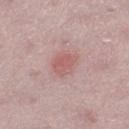Assessment:
Imaged during a routine full-body skin examination; the lesion was not biopsied and no histopathology is available.
Background:
The total-body-photography lesion software estimated a footprint of about 6.5 mm², an outline eccentricity of about 0.55 (0 = round, 1 = elongated), and a shape-asymmetry score of about 0.25 (0 = symmetric). The software also gave a mean CIELAB color near L≈58 a*≈24 b*≈22 and a lesion-to-skin contrast of about 5.5 (normalized; higher = more distinct). And it measured a border-irregularity rating of about 2/10, a color-variation rating of about 2/10, and peripheral color asymmetry of about 0.5. The software also gave an automated nevus-likeness rating near 30 out of 100 and a lesion-detection confidence of about 100/100. The lesion is on the right lower leg. Measured at roughly 3 mm in maximum diameter. The subject is a female in their mid-40s. Cropped from a whole-body photographic skin survey; the tile spans about 15 mm. Captured under white-light illumination.